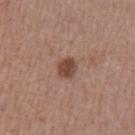lesion_size:
  long_diameter_mm_approx: 2.5
image:
  source: total-body photography crop
  field_of_view_mm: 15
site: left upper arm
patient:
  sex: male
  age_approx: 55